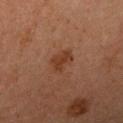biopsy status = total-body-photography surveillance lesion; no biopsy
location = the right upper arm
subject = female, aged approximately 60
illumination = cross-polarized
image = ~15 mm tile from a whole-body skin photo